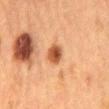No biopsy was performed on this lesion — it was imaged during a full skin examination and was not determined to be concerning.
The tile uses cross-polarized illumination.
Located on the mid back.
A close-up tile cropped from a whole-body skin photograph, about 15 mm across.
A female patient, roughly 60 years of age.